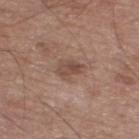biopsy status = catalogued during a skin exam; not biopsied
subject = male, roughly 65 years of age
tile lighting = white-light
body site = the leg
acquisition = total-body-photography crop, ~15 mm field of view